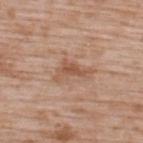Notes:
– follow-up — no biopsy performed (imaged during a skin exam)
– patient — male, aged around 50
– image source — ~15 mm crop, total-body skin-cancer survey
– automated metrics — border irregularity of about 6.5 on a 0–10 scale, a within-lesion color-variation index near 2.5/10, and radial color variation of about 0.5; a nevus-likeness score of about 0/100
– lesion size — ≈4.5 mm
– location — the upper back